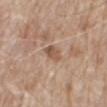Findings:
- biopsy status: total-body-photography surveillance lesion; no biopsy
- image-analysis metrics: a footprint of about 4 mm², an outline eccentricity of about 0.7 (0 = round, 1 = elongated), and a symmetry-axis asymmetry near 0.25
- patient: male, approximately 80 years of age
- imaging modality: 15 mm crop, total-body photography
- site: the mid back
- diameter: about 2.5 mm
- illumination: white-light